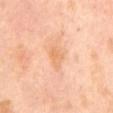Recorded during total-body skin imaging; not selected for excision or biopsy. A close-up tile cropped from a whole-body skin photograph, about 15 mm across. The subject is a male about 65 years old. This is a cross-polarized tile. An algorithmic analysis of the crop reported a lesion area of about 4 mm², an outline eccentricity of about 0.75 (0 = round, 1 = elongated), and two-axis asymmetry of about 0.3. And it measured a classifier nevus-likeness of about 0/100 and lesion-presence confidence of about 100/100.